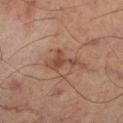workup = total-body-photography surveillance lesion; no biopsy
location = the leg
patient = male, aged 63 to 67
lesion size = ≈3.5 mm
imaging modality = ~15 mm tile from a whole-body skin photo